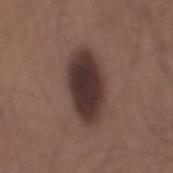The total-body-photography lesion software estimated a lesion area of about 20 mm², an outline eccentricity of about 0.85 (0 = round, 1 = elongated), and a symmetry-axis asymmetry near 0.1. The analysis additionally found a mean CIELAB color near L≈33 a*≈16 b*≈18, about 14 CIELAB-L* units darker than the surrounding skin, and a normalized lesion–skin contrast near 13. And it measured a nevus-likeness score of about 85/100 and a lesion-detection confidence of about 100/100.
Imaged with white-light lighting.
A lesion tile, about 15 mm wide, cut from a 3D total-body photograph.
A male subject, roughly 35 years of age.
The lesion's longest dimension is about 7 mm.
The lesion is on the mid back.